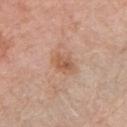Impression: The lesion was photographed on a routine skin check and not biopsied; there is no pathology result. Background: A female patient, in their 60s. A 15 mm close-up extracted from a 3D total-body photography capture. This is a white-light tile. The lesion is located on the left upper arm.